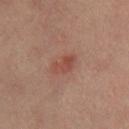workup: no biopsy performed (imaged during a skin exam).